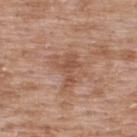{
  "biopsy_status": "not biopsied; imaged during a skin examination",
  "patient": {
    "sex": "male",
    "age_approx": 50
  },
  "lesion_size": {
    "long_diameter_mm_approx": 4.0
  },
  "image": {
    "source": "total-body photography crop",
    "field_of_view_mm": 15
  },
  "site": "upper back"
}